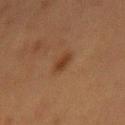Q: Was a biopsy performed?
A: total-body-photography surveillance lesion; no biopsy
Q: Where on the body is the lesion?
A: the mid back
Q: What did automated image analysis measure?
A: a lesion area of about 4.5 mm², an eccentricity of roughly 0.8, and a symmetry-axis asymmetry near 0.25; a border-irregularity rating of about 2.5/10, a color-variation rating of about 2/10, and radial color variation of about 0.5; an automated nevus-likeness rating near 100 out of 100 and a detector confidence of about 100 out of 100 that the crop contains a lesion
Q: How was this image acquired?
A: 15 mm crop, total-body photography
Q: Patient demographics?
A: female, approximately 50 years of age
Q: What is the lesion's diameter?
A: ≈3 mm
Q: What lighting was used for the tile?
A: cross-polarized illumination